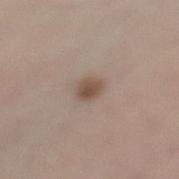biopsy status: catalogued during a skin exam; not biopsied
automated lesion analysis: an area of roughly 4 mm², a shape eccentricity near 0.7, and a symmetry-axis asymmetry near 0.25; an average lesion color of about L≈50 a*≈15 b*≈26 (CIELAB), about 11 CIELAB-L* units darker than the surrounding skin, and a lesion-to-skin contrast of about 8.5 (normalized; higher = more distinct); a color-variation rating of about 2.5/10; an automated nevus-likeness rating near 95 out of 100 and lesion-presence confidence of about 100/100
lesion size: ≈2.5 mm
tile lighting: white-light
subject: female, about 40 years old
image: total-body-photography crop, ~15 mm field of view
site: the left lower leg